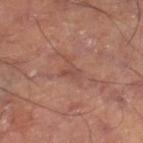biopsy status: total-body-photography surveillance lesion; no biopsy
TBP lesion metrics: border irregularity of about 6.5 on a 0–10 scale, a within-lesion color-variation index near 1.5/10, and a peripheral color-asymmetry measure near 0.5; a detector confidence of about 50 out of 100 that the crop contains a lesion
lighting: cross-polarized illumination
site: the right thigh
image: 15 mm crop, total-body photography